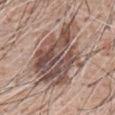A roughly 15 mm field-of-view crop from a total-body skin photograph. A male subject, aged approximately 60. Imaged with white-light lighting. Measured at roughly 10.5 mm in maximum diameter. The lesion is located on the front of the torso. Automated image analysis of the tile measured a lesion area of about 40 mm² and an eccentricity of roughly 0.8. The analysis additionally found a lesion color around L≈51 a*≈17 b*≈22 in CIELAB and about 13 CIELAB-L* units darker than the surrounding skin. The analysis additionally found a lesion-detection confidence of about 95/100.